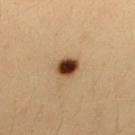This lesion was catalogued during total-body skin photography and was not selected for biopsy. The subject is a female aged approximately 35. The lesion is located on the upper back. The recorded lesion diameter is about 2.5 mm. A 15 mm crop from a total-body photograph taken for skin-cancer surveillance. Automated tile analysis of the lesion measured a lesion area of about 5.5 mm², an outline eccentricity of about 0.5 (0 = round, 1 = elongated), and a shape-asymmetry score of about 0.15 (0 = symmetric). It also reported a border-irregularity rating of about 1/10 and peripheral color asymmetry of about 1.5. It also reported a nevus-likeness score of about 100/100 and lesion-presence confidence of about 100/100. Imaged with cross-polarized lighting.